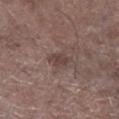Q: Is there a histopathology result?
A: catalogued during a skin exam; not biopsied
Q: What lighting was used for the tile?
A: white-light illumination
Q: What kind of image is this?
A: total-body-photography crop, ~15 mm field of view
Q: Who is the patient?
A: male, aged 68–72
Q: Lesion location?
A: the leg